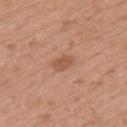This lesion was catalogued during total-body skin photography and was not selected for biopsy.
On the left upper arm.
A female patient approximately 50 years of age.
A 15 mm close-up extracted from a 3D total-body photography capture.